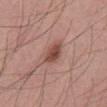Q: Was a biopsy performed?
A: total-body-photography surveillance lesion; no biopsy
Q: Patient demographics?
A: male, aged around 55
Q: What is the imaging modality?
A: ~15 mm tile from a whole-body skin photo
Q: What did automated image analysis measure?
A: an average lesion color of about L≈47 a*≈24 b*≈25 (CIELAB), roughly 13 lightness units darker than nearby skin, and a lesion-to-skin contrast of about 9 (normalized; higher = more distinct); border irregularity of about 2 on a 0–10 scale, internal color variation of about 2.5 on a 0–10 scale, and radial color variation of about 1
Q: Lesion size?
A: ~3 mm (longest diameter)
Q: Illumination type?
A: white-light
Q: What is the anatomic site?
A: the abdomen